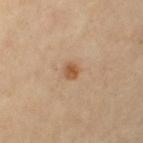Clinical impression:
Imaged during a routine full-body skin examination; the lesion was not biopsied and no histopathology is available.
Background:
The tile uses cross-polarized illumination. From the left upper arm. Longest diameter approximately 2 mm. Automated image analysis of the tile measured an area of roughly 3 mm², an eccentricity of roughly 0.5, and a symmetry-axis asymmetry near 0.15. And it measured a normalized lesion–skin contrast near 8.5. And it measured a border-irregularity rating of about 1/10, internal color variation of about 2 on a 0–10 scale, and peripheral color asymmetry of about 0.5. A female patient roughly 60 years of age. A roughly 15 mm field-of-view crop from a total-body skin photograph.